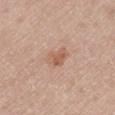The lesion was photographed on a routine skin check and not biopsied; there is no pathology result. The total-body-photography lesion software estimated a border-irregularity index near 3/10, a color-variation rating of about 2.5/10, and a peripheral color-asymmetry measure near 1. A male subject in their mid-70s. A 15 mm close-up extracted from a 3D total-body photography capture. Captured under white-light illumination. From the leg.